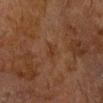This lesion was catalogued during total-body skin photography and was not selected for biopsy.
A 15 mm crop from a total-body photograph taken for skin-cancer surveillance.
Approximately 2.5 mm at its widest.
The total-body-photography lesion software estimated an area of roughly 3 mm², a shape eccentricity near 0.85, and a symmetry-axis asymmetry near 0.4. The analysis additionally found a lesion color around L≈29 a*≈17 b*≈27 in CIELAB, about 4 CIELAB-L* units darker than the surrounding skin, and a normalized lesion–skin contrast near 5. The analysis additionally found border irregularity of about 4 on a 0–10 scale and a color-variation rating of about 0.5/10.
Located on the head or neck.
The patient is a male approximately 75 years of age.
Captured under cross-polarized illumination.